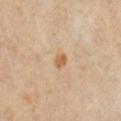Assessment:
The lesion was tiled from a total-body skin photograph and was not biopsied.
Context:
Longest diameter approximately 2 mm. Automated image analysis of the tile measured a lesion color around L≈62 a*≈20 b*≈38 in CIELAB, about 10 CIELAB-L* units darker than the surrounding skin, and a lesion-to-skin contrast of about 7.5 (normalized; higher = more distinct). Located on the right lower leg. A male patient about 55 years old. A region of skin cropped from a whole-body photographic capture, roughly 15 mm wide. This is a cross-polarized tile.